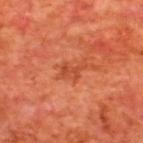Recorded during total-body skin imaging; not selected for excision or biopsy. An algorithmic analysis of the crop reported an eccentricity of roughly 0.8 and a symmetry-axis asymmetry near 0.35. The software also gave an average lesion color of about L≈44 a*≈32 b*≈37 (CIELAB), roughly 7 lightness units darker than nearby skin, and a lesion-to-skin contrast of about 5.5 (normalized; higher = more distinct). The software also gave an automated nevus-likeness rating near 0 out of 100 and a detector confidence of about 100 out of 100 that the crop contains a lesion. This image is a 15 mm lesion crop taken from a total-body photograph. The patient is a male aged approximately 65. The lesion is on the upper back. Captured under cross-polarized illumination. Measured at roughly 3 mm in maximum diameter.